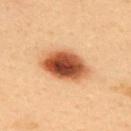Q: Was this lesion biopsied?
A: catalogued during a skin exam; not biopsied
Q: Patient demographics?
A: male, roughly 40 years of age
Q: What is the anatomic site?
A: the upper back
Q: How was the tile lit?
A: cross-polarized
Q: What is the lesion's diameter?
A: ~6 mm (longest diameter)
Q: What is the imaging modality?
A: 15 mm crop, total-body photography